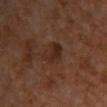follow-up: catalogued during a skin exam; not biopsied | tile lighting: cross-polarized illumination | patient: male, aged approximately 60 | imaging modality: 15 mm crop, total-body photography | site: the chest | automated lesion analysis: a lesion color around L≈25 a*≈18 b*≈23 in CIELAB and a lesion-to-skin contrast of about 7 (normalized; higher = more distinct); border irregularity of about 2.5 on a 0–10 scale, a within-lesion color-variation index near 3/10, and a peripheral color-asymmetry measure near 1.